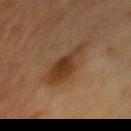Part of a total-body skin-imaging series; this lesion was reviewed on a skin check and was not flagged for biopsy. A female patient roughly 55 years of age. Cropped from a total-body skin-imaging series; the visible field is about 15 mm. On the chest.